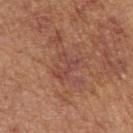No biopsy was performed on this lesion — it was imaged during a full skin examination and was not determined to be concerning. Located on the arm. A female subject in their mid- to late 70s. A 15 mm crop from a total-body photograph taken for skin-cancer surveillance.